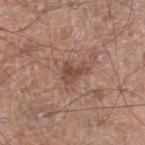workup: total-body-photography surveillance lesion; no biopsy
location: the leg
image: total-body-photography crop, ~15 mm field of view
patient: male, aged around 60
TBP lesion metrics: a lesion area of about 4.5 mm²